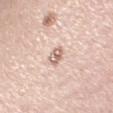Captured during whole-body skin photography for melanoma surveillance; the lesion was not biopsied. A 15 mm close-up tile from a total-body photography series done for melanoma screening. Located on the right upper arm. Approximately 2.5 mm at its widest. Automated tile analysis of the lesion measured an automated nevus-likeness rating near 0 out of 100 and a detector confidence of about 100 out of 100 that the crop contains a lesion. Captured under white-light illumination. The patient is a female about 60 years old.